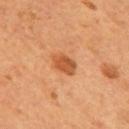The lesion was photographed on a routine skin check and not biopsied; there is no pathology result. A male subject aged around 55. The lesion is located on the mid back. This image is a 15 mm lesion crop taken from a total-body photograph.